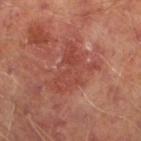Part of a total-body skin-imaging series; this lesion was reviewed on a skin check and was not flagged for biopsy. On the left lower leg. Imaged with cross-polarized lighting. An algorithmic analysis of the crop reported a footprint of about 15 mm², a shape eccentricity near 0.7, and two-axis asymmetry of about 0.45. It also reported internal color variation of about 3.5 on a 0–10 scale and a peripheral color-asymmetry measure near 1.5. A male patient, aged around 65. This image is a 15 mm lesion crop taken from a total-body photograph.